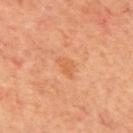{
  "biopsy_status": "not biopsied; imaged during a skin examination",
  "site": "back",
  "image": {
    "source": "total-body photography crop",
    "field_of_view_mm": 15
  },
  "lighting": "cross-polarized",
  "automated_metrics": {
    "vs_skin_darker_L": 6.0,
    "vs_skin_contrast_norm": 5.0,
    "border_irregularity_0_10": 3.5,
    "color_variation_0_10": 1.0,
    "nevus_likeness_0_100": 10,
    "lesion_detection_confidence_0_100": 100
  },
  "lesion_size": {
    "long_diameter_mm_approx": 2.5
  },
  "patient": {
    "sex": "male",
    "age_approx": 70
  }
}